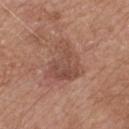notes: total-body-photography surveillance lesion; no biopsy.